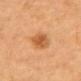Impression:
The lesion was tiled from a total-body skin photograph and was not biopsied.
Image and clinical context:
A close-up tile cropped from a whole-body skin photograph, about 15 mm across. The tile uses cross-polarized illumination. A female subject aged approximately 60. Located on the back.